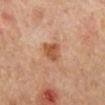Assessment:
This lesion was catalogued during total-body skin photography and was not selected for biopsy.
Background:
This is a cross-polarized tile. The lesion is on the right lower leg. A 15 mm close-up extracted from a 3D total-body photography capture. Approximately 2.5 mm at its widest. An algorithmic analysis of the crop reported a mean CIELAB color near L≈54 a*≈24 b*≈35, about 11 CIELAB-L* units darker than the surrounding skin, and a lesion-to-skin contrast of about 8 (normalized; higher = more distinct). The software also gave a border-irregularity rating of about 2.5/10, internal color variation of about 3 on a 0–10 scale, and peripheral color asymmetry of about 1. A female patient, aged 58–62.